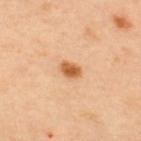site=the upper back; tile lighting=cross-polarized illumination; acquisition=total-body-photography crop, ~15 mm field of view; patient=male, aged 43 to 47.